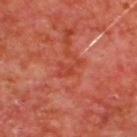Q: Was this lesion biopsied?
A: catalogued during a skin exam; not biopsied
Q: What is the anatomic site?
A: the back
Q: What is the lesion's diameter?
A: ≈3 mm
Q: What did automated image analysis measure?
A: a lesion area of about 4 mm² and a shape eccentricity near 0.85; a lesion color around L≈41 a*≈33 b*≈32 in CIELAB, a lesion–skin lightness drop of about 6, and a lesion-to-skin contrast of about 5.5 (normalized; higher = more distinct); border irregularity of about 6 on a 0–10 scale and radial color variation of about 0.5
Q: What kind of image is this?
A: ~15 mm crop, total-body skin-cancer survey
Q: What are the patient's age and sex?
A: male, approximately 65 years of age
Q: How was the tile lit?
A: cross-polarized illumination